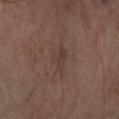No biopsy was performed on this lesion — it was imaged during a full skin examination and was not determined to be concerning. The recorded lesion diameter is about 3.5 mm. From the arm. This is a cross-polarized tile. A roughly 15 mm field-of-view crop from a total-body skin photograph. The subject is a male about 65 years old. An algorithmic analysis of the crop reported a mean CIELAB color near L≈35 a*≈13 b*≈20, a lesion–skin lightness drop of about 5, and a lesion-to-skin contrast of about 4.5 (normalized; higher = more distinct). The analysis additionally found a color-variation rating of about 2.5/10 and radial color variation of about 1.